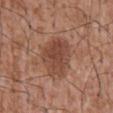Q: Was a biopsy performed?
A: no biopsy performed (imaged during a skin exam)
Q: What are the patient's age and sex?
A: male, approximately 45 years of age
Q: How large is the lesion?
A: about 5.5 mm
Q: What is the imaging modality?
A: ~15 mm tile from a whole-body skin photo
Q: Lesion location?
A: the abdomen